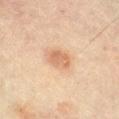{
  "biopsy_status": "not biopsied; imaged during a skin examination",
  "site": "right lower leg",
  "lesion_size": {
    "long_diameter_mm_approx": 3.0
  },
  "patient": {
    "sex": "female",
    "age_approx": 80
  },
  "image": {
    "source": "total-body photography crop",
    "field_of_view_mm": 15
  },
  "lighting": "cross-polarized"
}